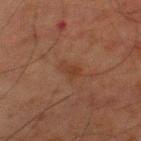  site: right thigh
  automated_metrics:
    area_mm2_approx: 3.5
    eccentricity: 0.9
    shape_asymmetry: 0.35
    cielab_L: 30
    cielab_a: 17
    cielab_b: 25
    vs_skin_contrast_norm: 5.5
    border_irregularity_0_10: 4.0
    color_variation_0_10: 1.0
    peripheral_color_asymmetry: 0.5
    lesion_detection_confidence_0_100: 100
  lesion_size:
    long_diameter_mm_approx: 3.0
  image:
    source: total-body photography crop
    field_of_view_mm: 15
  patient:
    sex: male
    age_approx: 80